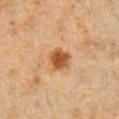<record>
  <biopsy_status>not biopsied; imaged during a skin examination</biopsy_status>
  <lesion_size>
    <long_diameter_mm_approx>3.0</long_diameter_mm_approx>
  </lesion_size>
  <patient>
    <sex>male</sex>
    <age_approx>50</age_approx>
  </patient>
  <lighting>cross-polarized</lighting>
  <site>chest</site>
  <automated_metrics>
    <area_mm2_approx>6.0</area_mm2_approx>
    <shape_asymmetry>0.2</shape_asymmetry>
    <border_irregularity_0_10>2.0</border_irregularity_0_10>
    <peripheral_color_asymmetry>1.0</peripheral_color_asymmetry>
  </automated_metrics>
  <image>
    <source>total-body photography crop</source>
    <field_of_view_mm>15</field_of_view_mm>
  </image>
</record>